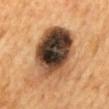| field | value |
|---|---|
| notes | catalogued during a skin exam; not biopsied |
| lighting | cross-polarized illumination |
| body site | the mid back |
| lesion diameter | ~7.5 mm (longest diameter) |
| subject | male, aged approximately 65 |
| imaging modality | total-body-photography crop, ~15 mm field of view |